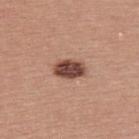The lesion was photographed on a routine skin check and not biopsied; there is no pathology result. A male subject in their 40s. A lesion tile, about 15 mm wide, cut from a 3D total-body photograph. On the upper back. Longest diameter approximately 3.5 mm.